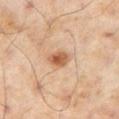Impression: Captured during whole-body skin photography for melanoma surveillance; the lesion was not biopsied. Acquisition and patient details: A 15 mm close-up tile from a total-body photography series done for melanoma screening. On the leg. Captured under cross-polarized illumination. The recorded lesion diameter is about 3 mm. The total-body-photography lesion software estimated an average lesion color of about L≈59 a*≈23 b*≈36 (CIELAB), roughly 13 lightness units darker than nearby skin, and a normalized lesion–skin contrast near 9. The patient is a male about 55 years old.